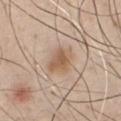Clinical impression: Captured during whole-body skin photography for melanoma surveillance; the lesion was not biopsied. Acquisition and patient details: The recorded lesion diameter is about 3.5 mm. Imaged with white-light lighting. Automated tile analysis of the lesion measured a lesion color around L≈58 a*≈17 b*≈31 in CIELAB and a lesion-to-skin contrast of about 7 (normalized; higher = more distinct). A male subject, aged 43–47. The lesion is located on the chest. A 15 mm crop from a total-body photograph taken for skin-cancer surveillance.